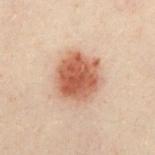Q: Was this lesion biopsied?
A: no biopsy performed (imaged during a skin exam)
Q: Lesion location?
A: the chest
Q: How was this image acquired?
A: total-body-photography crop, ~15 mm field of view
Q: What are the patient's age and sex?
A: male, in their 50s